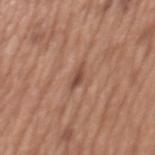Findings:
• automated metrics: a lesion–skin lightness drop of about 10; a border-irregularity index near 4/10, internal color variation of about 0.5 on a 0–10 scale, and peripheral color asymmetry of about 0; a nevus-likeness score of about 0/100 and a lesion-detection confidence of about 100/100
• patient: male, in their mid- to late 60s
• location: the mid back
• image: total-body-photography crop, ~15 mm field of view
• lighting: white-light illumination